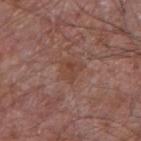Image and clinical context:
A male patient, aged around 75. An algorithmic analysis of the crop reported a mean CIELAB color near L≈43 a*≈21 b*≈26, roughly 6 lightness units darker than nearby skin, and a normalized border contrast of about 5.5. And it measured a border-irregularity rating of about 4/10 and a peripheral color-asymmetry measure near 1. From the right forearm. Longest diameter approximately 2.5 mm. A 15 mm close-up tile from a total-body photography series done for melanoma screening.